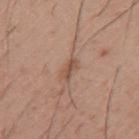Part of a total-body skin-imaging series; this lesion was reviewed on a skin check and was not flagged for biopsy.
From the upper back.
The patient is a male roughly 30 years of age.
The total-body-photography lesion software estimated a footprint of about 3 mm². And it measured about 9 CIELAB-L* units darker than the surrounding skin and a lesion-to-skin contrast of about 7 (normalized; higher = more distinct). The software also gave a border-irregularity rating of about 3.5/10, internal color variation of about 3.5 on a 0–10 scale, and radial color variation of about 1. It also reported a nevus-likeness score of about 0/100.
A close-up tile cropped from a whole-body skin photograph, about 15 mm across.
About 2.5 mm across.